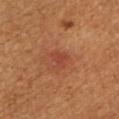– biopsy status · imaged on a skin check; not biopsied